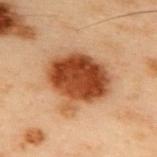follow-up: total-body-photography surveillance lesion; no biopsy
site: the upper back
subject: male, approximately 55 years of age
automated metrics: a footprint of about 28 mm², an outline eccentricity of about 0.45 (0 = round, 1 = elongated), and a shape-asymmetry score of about 0.25 (0 = symmetric); a nevus-likeness score of about 100/100 and a detector confidence of about 100 out of 100 that the crop contains a lesion
size: ~6 mm (longest diameter)
image source: ~15 mm tile from a whole-body skin photo
lighting: cross-polarized